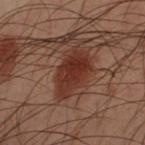workup = no biopsy performed (imaged during a skin exam) | image source = 15 mm crop, total-body photography | patient = male, approximately 50 years of age | anatomic site = the left forearm.